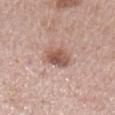imaging modality — ~15 mm crop, total-body skin-cancer survey | patient — female, aged around 50 | location — the left upper arm.